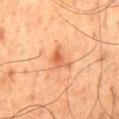Part of a total-body skin-imaging series; this lesion was reviewed on a skin check and was not flagged for biopsy.
The patient is a male in their 60s.
The lesion's longest dimension is about 3 mm.
Imaged with cross-polarized lighting.
This image is a 15 mm lesion crop taken from a total-body photograph.
Located on the mid back.
Automated image analysis of the tile measured an average lesion color of about L≈49 a*≈23 b*≈33 (CIELAB), about 8 CIELAB-L* units darker than the surrounding skin, and a lesion-to-skin contrast of about 6.5 (normalized; higher = more distinct).